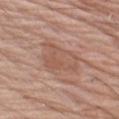workup: imaged on a skin check; not biopsied
image source: 15 mm crop, total-body photography
tile lighting: white-light illumination
anatomic site: the left upper arm
lesion size: about 6 mm
automated metrics: a border-irregularity index near 3.5/10, a color-variation rating of about 3/10, and peripheral color asymmetry of about 1
subject: male, approximately 70 years of age